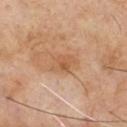Assessment:
Part of a total-body skin-imaging series; this lesion was reviewed on a skin check and was not flagged for biopsy.
Background:
A male subject, aged 68 to 72. The lesion is located on the chest. A 15 mm close-up extracted from a 3D total-body photography capture.